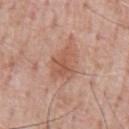notes=no biopsy performed (imaged during a skin exam) | lighting=white-light illumination | automated metrics=an area of roughly 9.5 mm² and two-axis asymmetry of about 0.3; a mean CIELAB color near L≈56 a*≈23 b*≈30, roughly 9 lightness units darker than nearby skin, and a normalized lesion–skin contrast near 6 | lesion diameter=~4 mm (longest diameter) | site=the front of the torso | subject=male, roughly 60 years of age | image source=~15 mm crop, total-body skin-cancer survey.